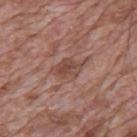This lesion was catalogued during total-body skin photography and was not selected for biopsy. Captured under white-light illumination. A male subject aged around 70. A region of skin cropped from a whole-body photographic capture, roughly 15 mm wide. Longest diameter approximately 4 mm. The total-body-photography lesion software estimated a symmetry-axis asymmetry near 0.3. The analysis additionally found a border-irregularity rating of about 3/10 and a color-variation rating of about 3.5/10. And it measured a classifier nevus-likeness of about 0/100. From the mid back.